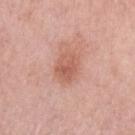• biopsy status: catalogued during a skin exam; not biopsied
• image: ~15 mm crop, total-body skin-cancer survey
• location: the arm
• size: ≈3.5 mm
• lighting: white-light
• subject: female, aged around 65
• automated metrics: a lesion area of about 8 mm²; an average lesion color of about L≈59 a*≈25 b*≈29 (CIELAB), roughly 9 lightness units darker than nearby skin, and a normalized border contrast of about 6.5; border irregularity of about 2 on a 0–10 scale, a within-lesion color-variation index near 4/10, and radial color variation of about 1.5; a classifier nevus-likeness of about 30/100